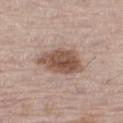| field | value |
|---|---|
| biopsy status | catalogued during a skin exam; not biopsied |
| body site | the right thigh |
| patient | male, aged 63 to 67 |
| acquisition | total-body-photography crop, ~15 mm field of view |